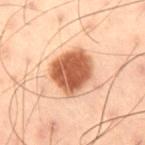Background:
The patient is a male aged 53–57. The lesion is located on the right thigh. A roughly 15 mm field-of-view crop from a total-body skin photograph. Captured under cross-polarized illumination. Approximately 4.5 mm at its widest.